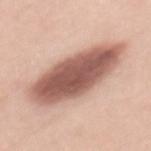Q: Was a biopsy performed?
A: catalogued during a skin exam; not biopsied
Q: Lesion location?
A: the mid back
Q: Automated lesion metrics?
A: a footprint of about 34 mm², an outline eccentricity of about 0.9 (0 = round, 1 = elongated), and two-axis asymmetry of about 0.15
Q: Patient demographics?
A: female, aged approximately 40
Q: What is the lesion's diameter?
A: ≈10 mm
Q: How was the tile lit?
A: white-light illumination
Q: What kind of image is this?
A: 15 mm crop, total-body photography